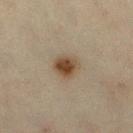workup: total-body-photography surveillance lesion; no biopsy.